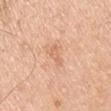Assessment: The lesion was tiled from a total-body skin photograph and was not biopsied. Background: The patient is a male about 55 years old. Located on the left upper arm. A lesion tile, about 15 mm wide, cut from a 3D total-body photograph. The tile uses white-light illumination. Automated tile analysis of the lesion measured an area of roughly 2.5 mm², an outline eccentricity of about 0.95 (0 = round, 1 = elongated), and a shape-asymmetry score of about 0.4 (0 = symmetric). The software also gave a mean CIELAB color near L≈67 a*≈23 b*≈36, about 7 CIELAB-L* units darker than the surrounding skin, and a normalized border contrast of about 5.